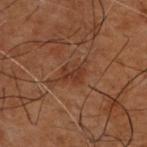Q: Was this lesion biopsied?
A: total-body-photography surveillance lesion; no biopsy
Q: Who is the patient?
A: male, in their 50s
Q: How large is the lesion?
A: ~2.5 mm (longest diameter)
Q: How was this image acquired?
A: 15 mm crop, total-body photography
Q: Illumination type?
A: cross-polarized illumination
Q: Lesion location?
A: the upper back